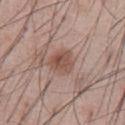biopsy status: imaged on a skin check; not biopsied | subject: male, aged approximately 55 | size: about 3 mm | automated metrics: an area of roughly 6.5 mm², an outline eccentricity of about 0.25 (0 = round, 1 = elongated), and a symmetry-axis asymmetry near 0.3; a within-lesion color-variation index near 4/10 and a peripheral color-asymmetry measure near 1.5; a classifier nevus-likeness of about 95/100 | acquisition: 15 mm crop, total-body photography | illumination: white-light illumination | site: the abdomen.